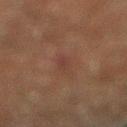biopsy status: imaged on a skin check; not biopsied
size: ≈3 mm
patient: male, in their mid- to late 60s
image: ~15 mm crop, total-body skin-cancer survey
illumination: cross-polarized
location: the right lower leg
automated lesion analysis: a lesion color around L≈31 a*≈18 b*≈21 in CIELAB, a lesion–skin lightness drop of about 5, and a normalized lesion–skin contrast near 5.5; a detector confidence of about 80 out of 100 that the crop contains a lesion